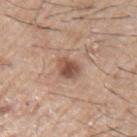  lighting: white-light
  patient:
    sex: male
    age_approx: 65
  image:
    source: total-body photography crop
    field_of_view_mm: 15
  automated_metrics:
    area_mm2_approx: 5.5
    eccentricity: 0.45
    shape_asymmetry: 0.2
    nevus_likeness_0_100: 90
    lesion_detection_confidence_0_100: 100
  site: left upper arm